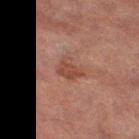| field | value |
|---|---|
| follow-up | catalogued during a skin exam; not biopsied |
| tile lighting | cross-polarized illumination |
| patient | female, aged 68–72 |
| anatomic site | the leg |
| imaging modality | ~15 mm crop, total-body skin-cancer survey |
| diameter | ≈3 mm |